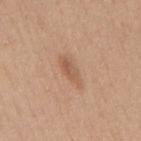biopsy_status: not biopsied; imaged during a skin examination
patient:
  sex: male
  age_approx: 30
lighting: white-light
image:
  source: total-body photography crop
  field_of_view_mm: 15
site: back
lesion_size:
  long_diameter_mm_approx: 4.5